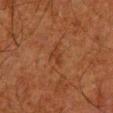<lesion>
<biopsy_status>not biopsied; imaged during a skin examination</biopsy_status>
<site>left lower leg</site>
<automated_metrics>
  <area_mm2_approx>2.0</area_mm2_approx>
  <eccentricity>0.9</eccentricity>
  <shape_asymmetry>0.4</shape_asymmetry>
  <cielab_L>29</cielab_L>
  <cielab_a>20</cielab_a>
  <cielab_b>28</cielab_b>
  <border_irregularity_0_10>4.5</border_irregularity_0_10>
  <color_variation_0_10>0.0</color_variation_0_10>
  <peripheral_color_asymmetry>0.0</peripheral_color_asymmetry>
</automated_metrics>
<lighting>cross-polarized</lighting>
<patient>
  <sex>male</sex>
  <age_approx>80</age_approx>
</patient>
<image>
  <source>total-body photography crop</source>
  <field_of_view_mm>15</field_of_view_mm>
</image>
</lesion>